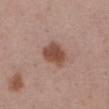Impression: No biopsy was performed on this lesion — it was imaged during a full skin examination and was not determined to be concerning. Context: Measured at roughly 4 mm in maximum diameter. A female subject, aged approximately 55. Captured under white-light illumination. The lesion is on the front of the torso. Cropped from a whole-body photographic skin survey; the tile spans about 15 mm. The lesion-visualizer software estimated a mean CIELAB color near L≈49 a*≈21 b*≈27, about 12 CIELAB-L* units darker than the surrounding skin, and a normalized lesion–skin contrast near 9.